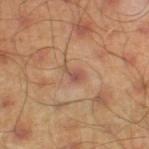body site=the left thigh; subject=male, in their mid- to late 40s; imaging modality=15 mm crop, total-body photography; illumination=cross-polarized illumination; lesion diameter=≈2.5 mm.